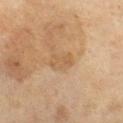Part of a total-body skin-imaging series; this lesion was reviewed on a skin check and was not flagged for biopsy.
A close-up tile cropped from a whole-body skin photograph, about 15 mm across.
Longest diameter approximately 3 mm.
The total-body-photography lesion software estimated a mean CIELAB color near L≈50 a*≈16 b*≈33, roughly 5 lightness units darker than nearby skin, and a normalized border contrast of about 4.5.
On the right lower leg.
Imaged with cross-polarized lighting.
The subject is a female aged around 70.